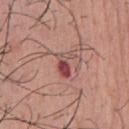Clinical impression: Imaged during a routine full-body skin examination; the lesion was not biopsied and no histopathology is available. Acquisition and patient details: Imaged with white-light lighting. A 15 mm crop from a total-body photograph taken for skin-cancer surveillance. A male subject, aged 53 to 57. The recorded lesion diameter is about 3.5 mm. The lesion is located on the chest.